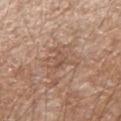Clinical impression:
Recorded during total-body skin imaging; not selected for excision or biopsy.
Background:
Automated tile analysis of the lesion measured an area of roughly 3.5 mm², a shape eccentricity near 0.8, and a symmetry-axis asymmetry near 0.45. A male subject, about 55 years old. From the arm. This is a white-light tile. Cropped from a total-body skin-imaging series; the visible field is about 15 mm.